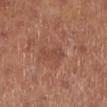An algorithmic analysis of the crop reported a mean CIELAB color near L≈46 a*≈25 b*≈28, about 7 CIELAB-L* units darker than the surrounding skin, and a normalized lesion–skin contrast near 5. The software also gave a border-irregularity rating of about 6/10 and peripheral color asymmetry of about 0.5. It also reported an automated nevus-likeness rating near 0 out of 100. Measured at roughly 3.5 mm in maximum diameter. A 15 mm close-up tile from a total-body photography series done for melanoma screening. On the left lower leg. A female subject, in their mid-60s. Imaged with white-light lighting.